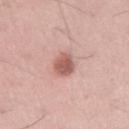Assessment: The lesion was tiled from a total-body skin photograph and was not biopsied. Acquisition and patient details: The patient is a male about 35 years old. The lesion's longest dimension is about 3.5 mm. Imaged with white-light lighting. The lesion is on the right upper arm. A roughly 15 mm field-of-view crop from a total-body skin photograph.